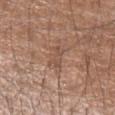Impression:
This lesion was catalogued during total-body skin photography and was not selected for biopsy.
Background:
The lesion is located on the arm. Approximately 3 mm at its widest. This is a white-light tile. Cropped from a whole-body photographic skin survey; the tile spans about 15 mm. The subject is a male aged 53–57.